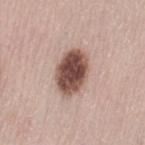follow-up = no biopsy performed (imaged during a skin exam) | image source = ~15 mm tile from a whole-body skin photo | location = the left thigh | patient = female, in their mid- to late 60s.